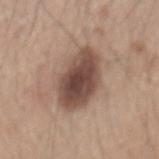biopsy status: no biopsy performed (imaged during a skin exam) | automated metrics: a lesion area of about 22 mm², an outline eccentricity of about 0.75 (0 = round, 1 = elongated), and a shape-asymmetry score of about 0.15 (0 = symmetric); a mean CIELAB color near L≈48 a*≈18 b*≈24, roughly 15 lightness units darker than nearby skin, and a lesion-to-skin contrast of about 10.5 (normalized; higher = more distinct); a nevus-likeness score of about 85/100 and lesion-presence confidence of about 100/100 | image source: 15 mm crop, total-body photography | patient: male, aged around 45 | diameter: about 6.5 mm | anatomic site: the mid back | illumination: white-light.